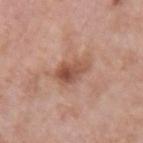Image and clinical context: The tile uses white-light illumination. A 15 mm close-up tile from a total-body photography series done for melanoma screening. On the right forearm. The subject is a female approximately 70 years of age. Longest diameter approximately 4.5 mm.